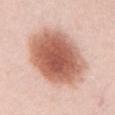notes=catalogued during a skin exam; not biopsied | body site=the chest | diameter=≈8 mm | tile lighting=white-light illumination | image source=~15 mm tile from a whole-body skin photo | subject=male, aged 23–27.